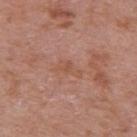| key | value |
|---|---|
| workup | imaged on a skin check; not biopsied |
| automated lesion analysis | a lesion area of about 3.5 mm², an eccentricity of roughly 0.85, and a shape-asymmetry score of about 0.4 (0 = symmetric); roughly 5 lightness units darker than nearby skin and a normalized border contrast of about 5; a border-irregularity index near 4.5/10 and internal color variation of about 1.5 on a 0–10 scale; an automated nevus-likeness rating near 0 out of 100 and a lesion-detection confidence of about 100/100 |
| image | 15 mm crop, total-body photography |
| site | the arm |
| lesion size | about 3 mm |
| patient | male, aged 68–72 |